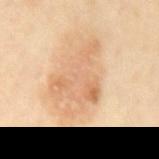Findings:
- biopsy status: total-body-photography surveillance lesion; no biopsy
- lighting: cross-polarized
- location: the back
- image: 15 mm crop, total-body photography
- subject: male, in their mid- to late 60s